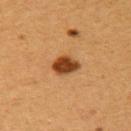lighting: cross-polarized illumination; subject: female, about 40 years old; acquisition: 15 mm crop, total-body photography; site: the upper back; lesion diameter: ≈3 mm.